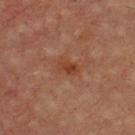This lesion was catalogued during total-body skin photography and was not selected for biopsy. A close-up tile cropped from a whole-body skin photograph, about 15 mm across. The lesion's longest dimension is about 2.5 mm. The subject is a male roughly 65 years of age. On the upper back.